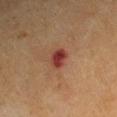– biopsy status: no biopsy performed (imaged during a skin exam)
– acquisition: 15 mm crop, total-body photography
– lesion diameter: ≈3 mm
– patient: male, aged approximately 65
– automated metrics: an area of roughly 4.5 mm² and a shape eccentricity near 0.7; a lesion–skin lightness drop of about 12 and a lesion-to-skin contrast of about 11 (normalized; higher = more distinct); a border-irregularity index near 2/10, a within-lesion color-variation index near 3.5/10, and peripheral color asymmetry of about 1; a nevus-likeness score of about 0/100 and lesion-presence confidence of about 100/100
– location: the left upper arm